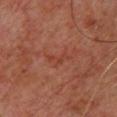Impression:
Part of a total-body skin-imaging series; this lesion was reviewed on a skin check and was not flagged for biopsy.
Context:
From the upper back. A close-up tile cropped from a whole-body skin photograph, about 15 mm across. The subject is a male about 60 years old. Longest diameter approximately 3 mm.